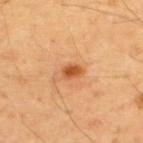This lesion was catalogued during total-body skin photography and was not selected for biopsy. Cropped from a whole-body photographic skin survey; the tile spans about 15 mm. A male patient aged 53 to 57. Captured under cross-polarized illumination. The lesion is on the back.